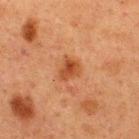The lesion was photographed on a routine skin check and not biopsied; there is no pathology result.
The total-body-photography lesion software estimated a shape eccentricity near 0.55 and a symmetry-axis asymmetry near 0.3. The analysis additionally found a lesion color around L≈39 a*≈23 b*≈33 in CIELAB, about 9 CIELAB-L* units darker than the surrounding skin, and a normalized border contrast of about 8. And it measured border irregularity of about 2.5 on a 0–10 scale, internal color variation of about 4 on a 0–10 scale, and a peripheral color-asymmetry measure near 1. And it measured a nevus-likeness score of about 80/100.
Longest diameter approximately 2.5 mm.
The tile uses cross-polarized illumination.
Located on the back.
A close-up tile cropped from a whole-body skin photograph, about 15 mm across.
A male subject aged 58–62.